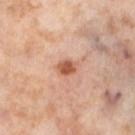Imaged during a routine full-body skin examination; the lesion was not biopsied and no histopathology is available. A roughly 15 mm field-of-view crop from a total-body skin photograph. Longest diameter approximately 2.5 mm. Captured under cross-polarized illumination. An algorithmic analysis of the crop reported a lesion area of about 4 mm², an eccentricity of roughly 0.7, and two-axis asymmetry of about 0.2. And it measured a color-variation rating of about 2.5/10 and a peripheral color-asymmetry measure near 1. A female subject aged approximately 55. Located on the left lower leg.